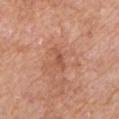• biopsy status · catalogued during a skin exam; not biopsied
• location · the arm
• subject · male, about 60 years old
• image source · total-body-photography crop, ~15 mm field of view
• tile lighting · white-light illumination